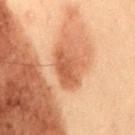No biopsy was performed on this lesion — it was imaged during a full skin examination and was not determined to be concerning.
On the lower back.
The tile uses cross-polarized illumination.
Approximately 4.5 mm at its widest.
A male patient in their mid-50s.
A region of skin cropped from a whole-body photographic capture, roughly 15 mm wide.